A male subject, aged 68 to 72. Captured under white-light illumination. On the arm. Cropped from a whole-body photographic skin survey; the tile spans about 15 mm: 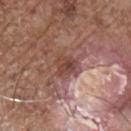{
  "diagnosis": {
    "histopathology": "invasive squamous cell carcinoma",
    "malignancy": "malignant",
    "taxonomic_path": [
      "Malignant",
      "Malignant epidermal proliferations",
      "Squamous cell carcinoma, Invasive"
    ]
  }
}